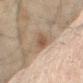Assessment: This lesion was catalogued during total-body skin photography and was not selected for biopsy. Clinical summary: A region of skin cropped from a whole-body photographic capture, roughly 15 mm wide. Imaged with cross-polarized lighting. A male subject roughly 45 years of age. On the left forearm. The lesion's longest dimension is about 3.5 mm.